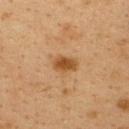Imaged during a routine full-body skin examination; the lesion was not biopsied and no histopathology is available. Captured under cross-polarized illumination. A female subject, about 40 years old. An algorithmic analysis of the crop reported a border-irregularity index near 2/10, internal color variation of about 2 on a 0–10 scale, and peripheral color asymmetry of about 0.5. The software also gave a nevus-likeness score of about 90/100 and a detector confidence of about 100 out of 100 that the crop contains a lesion. Located on the upper back. Measured at roughly 3 mm in maximum diameter. A 15 mm close-up extracted from a 3D total-body photography capture.